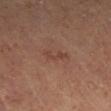Impression: The lesion was photographed on a routine skin check and not biopsied; there is no pathology result. Acquisition and patient details: The lesion is located on the right lower leg. A close-up tile cropped from a whole-body skin photograph, about 15 mm across. About 3 mm across. Imaged with cross-polarized lighting. A female subject, approximately 40 years of age. An algorithmic analysis of the crop reported a mean CIELAB color near L≈41 a*≈21 b*≈26 and a normalized border contrast of about 5. The analysis additionally found a nevus-likeness score of about 0/100 and lesion-presence confidence of about 100/100.